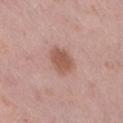Findings:
– notes: catalogued during a skin exam; not biopsied
– diameter: ~3.5 mm (longest diameter)
– subject: female, in their 40s
– image source: total-body-photography crop, ~15 mm field of view
– site: the right thigh
– tile lighting: white-light illumination